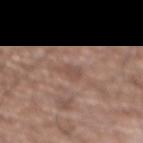Clinical impression: No biopsy was performed on this lesion — it was imaged during a full skin examination and was not determined to be concerning. Image and clinical context: The patient is a male in their mid- to late 70s. An algorithmic analysis of the crop reported a lesion color around L≈50 a*≈18 b*≈24 in CIELAB, about 6 CIELAB-L* units darker than the surrounding skin, and a normalized border contrast of about 5. The software also gave a color-variation rating of about 2/10 and radial color variation of about 0.5. Approximately 2.5 mm at its widest. Captured under white-light illumination. On the abdomen. A 15 mm close-up tile from a total-body photography series done for melanoma screening.